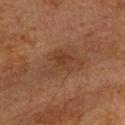The lesion is on the head or neck. A close-up tile cropped from a whole-body skin photograph, about 15 mm across. A male patient, roughly 65 years of age. Automated image analysis of the tile measured a border-irregularity index near 5/10 and a within-lesion color-variation index near 3.5/10. The software also gave a nevus-likeness score of about 5/100. Imaged with cross-polarized lighting. About 5.5 mm across.